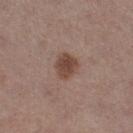Part of a total-body skin-imaging series; this lesion was reviewed on a skin check and was not flagged for biopsy.